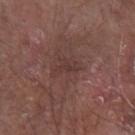<tbp_lesion>
  <biopsy_status>not biopsied; imaged during a skin examination</biopsy_status>
  <patient>
    <sex>male</sex>
    <age_approx>80</age_approx>
  </patient>
  <site>left forearm</site>
  <lesion_size>
    <long_diameter_mm_approx>2.5</long_diameter_mm_approx>
  </lesion_size>
  <image>
    <source>total-body photography crop</source>
    <field_of_view_mm>15</field_of_view_mm>
  </image>
  <automated_metrics>
    <nevus_likeness_0_100>0</nevus_likeness_0_100>
  </automated_metrics>
  <lighting>white-light</lighting>
</tbp_lesion>